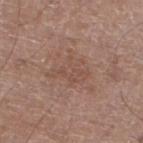notes = imaged on a skin check; not biopsied
automated lesion analysis = an eccentricity of roughly 0.7 and two-axis asymmetry of about 0.55
body site = the left lower leg
subject = male, aged around 60
lighting = white-light
imaging modality = 15 mm crop, total-body photography
size = ~4 mm (longest diameter)